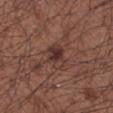Acquisition and patient details:
A region of skin cropped from a whole-body photographic capture, roughly 15 mm wide. On the left forearm. A male patient, about 55 years old. The lesion's longest dimension is about 3 mm. An algorithmic analysis of the crop reported a border-irregularity index near 2.5/10 and peripheral color asymmetry of about 1.5.